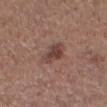Notes:
• image-analysis metrics — a mean CIELAB color near L≈42 a*≈19 b*≈22, a lesion–skin lightness drop of about 10, and a normalized border contrast of about 8; an automated nevus-likeness rating near 55 out of 100 and a lesion-detection confidence of about 100/100
• acquisition — ~15 mm crop, total-body skin-cancer survey
• site — the head or neck
• patient — male, aged around 35